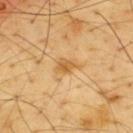Captured during whole-body skin photography for melanoma surveillance; the lesion was not biopsied.
On the upper back.
The total-body-photography lesion software estimated internal color variation of about 3 on a 0–10 scale. The software also gave an automated nevus-likeness rating near 5 out of 100 and lesion-presence confidence of about 100/100.
Captured under cross-polarized illumination.
Measured at roughly 3 mm in maximum diameter.
The subject is a male roughly 65 years of age.
A 15 mm close-up tile from a total-body photography series done for melanoma screening.